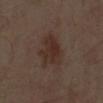Q: Was a biopsy performed?
A: imaged on a skin check; not biopsied
Q: Where on the body is the lesion?
A: the left arm
Q: Illumination type?
A: cross-polarized
Q: Who is the patient?
A: male, aged around 50
Q: Lesion size?
A: about 4.5 mm
Q: What is the imaging modality?
A: total-body-photography crop, ~15 mm field of view